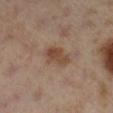follow-up: catalogued during a skin exam; not biopsied | acquisition: total-body-photography crop, ~15 mm field of view | tile lighting: cross-polarized illumination | location: the right lower leg | patient: female, about 40 years old | lesion size: ~4 mm (longest diameter).